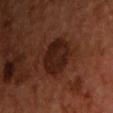Notes:
• workup: total-body-photography surveillance lesion; no biopsy
• illumination: cross-polarized
• site: the chest
• diameter: ~4.5 mm (longest diameter)
• automated lesion analysis: a lesion area of about 16 mm², a shape eccentricity near 0.25, and a symmetry-axis asymmetry near 0.15; roughly 8 lightness units darker than nearby skin and a normalized border contrast of about 9.5; border irregularity of about 2 on a 0–10 scale, a within-lesion color-variation index near 2.5/10, and radial color variation of about 1; a classifier nevus-likeness of about 15/100 and lesion-presence confidence of about 100/100
• imaging modality: ~15 mm crop, total-body skin-cancer survey
• subject: male, aged approximately 55